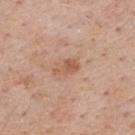Notes:
– follow-up: no biopsy performed (imaged during a skin exam)
– image source: ~15 mm crop, total-body skin-cancer survey
– subject: male, about 60 years old
– body site: the upper back